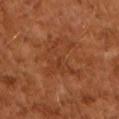Q: Was a biopsy performed?
A: catalogued during a skin exam; not biopsied
Q: What is the imaging modality?
A: ~15 mm tile from a whole-body skin photo
Q: Who is the patient?
A: male, in their mid-60s
Q: How was the tile lit?
A: cross-polarized illumination